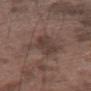No biopsy was performed on this lesion — it was imaged during a full skin examination and was not determined to be concerning. A male patient aged 73 to 77. This image is a 15 mm lesion crop taken from a total-body photograph. On the right thigh.